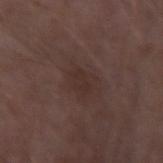Q: Is there a histopathology result?
A: catalogued during a skin exam; not biopsied
Q: How was this image acquired?
A: ~15 mm crop, total-body skin-cancer survey
Q: Patient demographics?
A: male, about 75 years old
Q: What is the anatomic site?
A: the right forearm
Q: How was the tile lit?
A: white-light illumination